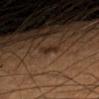Captured during whole-body skin photography for melanoma surveillance; the lesion was not biopsied. The total-body-photography lesion software estimated an area of roughly 2 mm² and two-axis asymmetry of about 0.35. It also reported a lesion color around L≈25 a*≈16 b*≈24 in CIELAB, roughly 8 lightness units darker than nearby skin, and a normalized border contrast of about 8.5. A male patient, in their mid- to late 60s. Cropped from a total-body skin-imaging series; the visible field is about 15 mm. This is a cross-polarized tile. On the left forearm. Longest diameter approximately 2 mm.